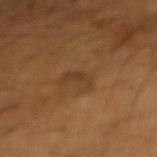tile lighting: cross-polarized illumination | imaging modality: 15 mm crop, total-body photography | subject: male, in their mid-60s | size: ~3 mm (longest diameter) | location: the right forearm.